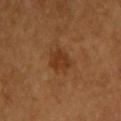Assessment:
This lesion was catalogued during total-body skin photography and was not selected for biopsy.
Acquisition and patient details:
The lesion is located on the upper back. About 3 mm across. A male patient, aged around 65. Automated image analysis of the tile measured an eccentricity of roughly 0.55 and a symmetry-axis asymmetry near 0.25. It also reported a detector confidence of about 100 out of 100 that the crop contains a lesion. A lesion tile, about 15 mm wide, cut from a 3D total-body photograph.